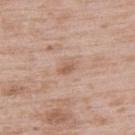Q: Patient demographics?
A: male, roughly 50 years of age
Q: Illumination type?
A: white-light
Q: Lesion location?
A: the upper back
Q: What is the imaging modality?
A: ~15 mm tile from a whole-body skin photo